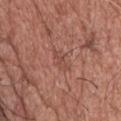notes: imaged on a skin check; not biopsied
lesion size: about 3.5 mm
subject: male, in their mid- to late 50s
imaging modality: total-body-photography crop, ~15 mm field of view
location: the back
automated lesion analysis: a within-lesion color-variation index near 1/10 and radial color variation of about 0.5
lighting: white-light illumination